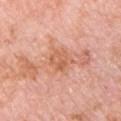Q: Was a biopsy performed?
A: catalogued during a skin exam; not biopsied
Q: What kind of image is this?
A: 15 mm crop, total-body photography
Q: What is the anatomic site?
A: the chest
Q: Illumination type?
A: white-light illumination
Q: What are the patient's age and sex?
A: male, aged 78–82